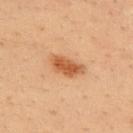Q: Was this lesion biopsied?
A: total-body-photography surveillance lesion; no biopsy
Q: Lesion location?
A: the upper back
Q: Patient demographics?
A: male, in their mid-30s
Q: How was this image acquired?
A: ~15 mm tile from a whole-body skin photo
Q: What is the lesion's diameter?
A: ≈4.5 mm